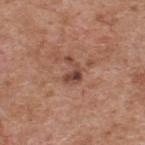Part of a total-body skin-imaging series; this lesion was reviewed on a skin check and was not flagged for biopsy. A male subject roughly 60 years of age. The recorded lesion diameter is about 3 mm. Located on the upper back. Automated image analysis of the tile measured a footprint of about 4 mm², an eccentricity of roughly 0.75, and a symmetry-axis asymmetry near 0.6. The software also gave a nevus-likeness score of about 0/100 and a detector confidence of about 100 out of 100 that the crop contains a lesion. A 15 mm close-up extracted from a 3D total-body photography capture. This is a white-light tile.